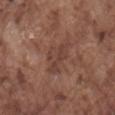Impression: The lesion was photographed on a routine skin check and not biopsied; there is no pathology result. Background: Captured under white-light illumination. A 15 mm close-up extracted from a 3D total-body photography capture. The recorded lesion diameter is about 4.5 mm. A male subject in their mid- to late 70s. Automated tile analysis of the lesion measured a footprint of about 7.5 mm², a shape eccentricity near 0.8, and a symmetry-axis asymmetry near 0.45. The software also gave a mean CIELAB color near L≈41 a*≈20 b*≈24, a lesion–skin lightness drop of about 6, and a normalized lesion–skin contrast near 5.5. It also reported border irregularity of about 5.5 on a 0–10 scale and a peripheral color-asymmetry measure near 1. The analysis additionally found a nevus-likeness score of about 0/100 and lesion-presence confidence of about 85/100. Located on the mid back.